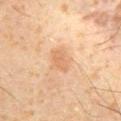Image and clinical context:
A male patient, aged approximately 60. Imaged with cross-polarized lighting. Automated tile analysis of the lesion measured an area of roughly 4.5 mm², an eccentricity of roughly 0.75, and a shape-asymmetry score of about 0.2 (0 = symmetric). And it measured a mean CIELAB color near L≈55 a*≈19 b*≈32, roughly 7 lightness units darker than nearby skin, and a normalized border contrast of about 5. And it measured a border-irregularity rating of about 2/10, a color-variation rating of about 1.5/10, and radial color variation of about 0.5. From the chest. Measured at roughly 2.5 mm in maximum diameter. A 15 mm close-up tile from a total-body photography series done for melanoma screening.